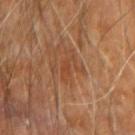| field | value |
|---|---|
| workup | imaged on a skin check; not biopsied |
| lesion size | ≈4 mm |
| automated metrics | a lesion color around L≈42 a*≈22 b*≈33 in CIELAB and a lesion–skin lightness drop of about 5; a border-irregularity index near 6/10, internal color variation of about 0.5 on a 0–10 scale, and peripheral color asymmetry of about 0 |
| patient | male, aged 58–62 |
| image | total-body-photography crop, ~15 mm field of view |
| illumination | cross-polarized |
| body site | the arm |